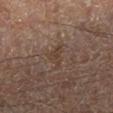notes: imaged on a skin check; not biopsied | image: 15 mm crop, total-body photography | automated lesion analysis: an outline eccentricity of about 0.75 (0 = round, 1 = elongated) and a shape-asymmetry score of about 0.55 (0 = symmetric); a border-irregularity rating of about 5.5/10, a color-variation rating of about 1.5/10, and a peripheral color-asymmetry measure near 0.5; a nevus-likeness score of about 0/100 and a lesion-detection confidence of about 90/100 | tile lighting: cross-polarized | location: the right lower leg | subject: male, aged 63 to 67 | lesion diameter: ~3.5 mm (longest diameter).